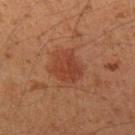No biopsy was performed on this lesion — it was imaged during a full skin examination and was not determined to be concerning.
Located on the left upper arm.
Automated image analysis of the tile measured an area of roughly 10 mm², a shape eccentricity near 0.45, and a shape-asymmetry score of about 0.2 (0 = symmetric). The software also gave an average lesion color of about L≈37 a*≈24 b*≈30 (CIELAB), about 7 CIELAB-L* units darker than the surrounding skin, and a lesion-to-skin contrast of about 6.5 (normalized; higher = more distinct). The software also gave a classifier nevus-likeness of about 90/100 and lesion-presence confidence of about 100/100.
Imaged with cross-polarized lighting.
The lesion's longest dimension is about 4 mm.
A region of skin cropped from a whole-body photographic capture, roughly 15 mm wide.
A male patient about 50 years old.